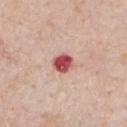notes — catalogued during a skin exam; not biopsied | image-analysis metrics — an area of roughly 5 mm² and a shape eccentricity near 0.5; an average lesion color of about L≈52 a*≈35 b*≈24 (CIELAB), roughly 20 lightness units darker than nearby skin, and a lesion-to-skin contrast of about 12.5 (normalized; higher = more distinct) | image source — total-body-photography crop, ~15 mm field of view | lesion size — ~2.5 mm (longest diameter) | subject — male, aged approximately 60 | illumination — white-light illumination | location — the chest.